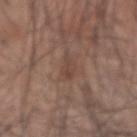Clinical impression: The lesion was tiled from a total-body skin photograph and was not biopsied. Acquisition and patient details: A male subject, roughly 45 years of age. Captured under white-light illumination. About 3.5 mm across. Cropped from a total-body skin-imaging series; the visible field is about 15 mm. The lesion is located on the right forearm.